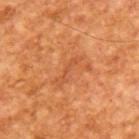workup = imaged on a skin check; not biopsied
patient = male, aged 63–67
lesion diameter = ~4 mm (longest diameter)
illumination = cross-polarized illumination
acquisition = 15 mm crop, total-body photography